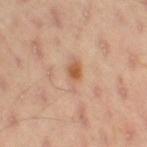Recorded during total-body skin imaging; not selected for excision or biopsy.
A male patient, about 55 years old.
The tile uses cross-polarized illumination.
An algorithmic analysis of the crop reported a lesion color around L≈61 a*≈20 b*≈32 in CIELAB and a normalized lesion–skin contrast near 5. It also reported border irregularity of about 2.5 on a 0–10 scale, a within-lesion color-variation index near 7/10, and a peripheral color-asymmetry measure near 2. It also reported a classifier nevus-likeness of about 65/100 and a detector confidence of about 100 out of 100 that the crop contains a lesion.
Cropped from a total-body skin-imaging series; the visible field is about 15 mm.
Located on the right thigh.